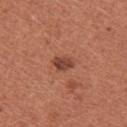Automated tile analysis of the lesion measured a footprint of about 4 mm². The analysis additionally found a mean CIELAB color near L≈44 a*≈26 b*≈29, a lesion–skin lightness drop of about 11, and a normalized border contrast of about 8.5.
A 15 mm close-up extracted from a 3D total-body photography capture.
The lesion is located on the left upper arm.
A female subject aged around 35.
About 3 mm across.
This is a white-light tile.